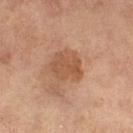| field | value |
|---|---|
| workup | imaged on a skin check; not biopsied |
| anatomic site | the right thigh |
| lesion diameter | ~4 mm (longest diameter) |
| subject | female, aged 58 to 62 |
| imaging modality | 15 mm crop, total-body photography |
| tile lighting | cross-polarized |